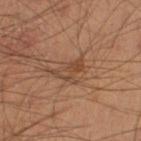{"biopsy_status": "not biopsied; imaged during a skin examination", "automated_metrics": {"area_mm2_approx": 5.0, "shape_asymmetry": 0.55, "cielab_L": 45, "cielab_a": 19, "cielab_b": 31, "vs_skin_darker_L": 7.0, "vs_skin_contrast_norm": 6.0, "nevus_likeness_0_100": 5}, "lighting": "cross-polarized", "site": "leg", "image": {"source": "total-body photography crop", "field_of_view_mm": 15}, "lesion_size": {"long_diameter_mm_approx": 3.5}, "patient": {"sex": "male", "age_approx": 40}}